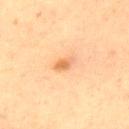Captured during whole-body skin photography for melanoma surveillance; the lesion was not biopsied. The lesion's longest dimension is about 2.5 mm. The lesion-visualizer software estimated an area of roughly 3 mm² and a shape eccentricity near 0.85. The software also gave roughly 9 lightness units darker than nearby skin and a normalized border contrast of about 7. On the front of the torso. A 15 mm close-up extracted from a 3D total-body photography capture. A male subject about 65 years old. This is a cross-polarized tile.